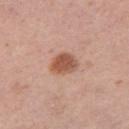Q: Was this lesion biopsied?
A: catalogued during a skin exam; not biopsied
Q: What is the imaging modality?
A: 15 mm crop, total-body photography
Q: Who is the patient?
A: female, aged approximately 40
Q: What is the anatomic site?
A: the leg
Q: Illumination type?
A: white-light illumination
Q: What is the lesion's diameter?
A: about 3.5 mm
Q: Automated lesion metrics?
A: an area of roughly 7.5 mm² and an outline eccentricity of about 0.55 (0 = round, 1 = elongated); a lesion color around L≈54 a*≈23 b*≈30 in CIELAB, roughly 13 lightness units darker than nearby skin, and a lesion-to-skin contrast of about 9 (normalized; higher = more distinct); a nevus-likeness score of about 100/100 and a lesion-detection confidence of about 100/100